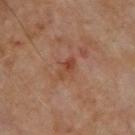Impression: The lesion was photographed on a routine skin check and not biopsied; there is no pathology result. Acquisition and patient details: The lesion is located on the chest. A roughly 15 mm field-of-view crop from a total-body skin photograph. A male patient in their mid- to late 50s.